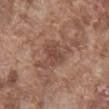Q: Was a biopsy performed?
A: no biopsy performed (imaged during a skin exam)
Q: What is the anatomic site?
A: the chest
Q: Patient demographics?
A: male, about 75 years old
Q: What is the imaging modality?
A: total-body-photography crop, ~15 mm field of view
Q: Lesion size?
A: about 5.5 mm
Q: What did automated image analysis measure?
A: a lesion area of about 12 mm², a shape eccentricity near 0.6, and a symmetry-axis asymmetry near 0.4; an average lesion color of about L≈47 a*≈20 b*≈26 (CIELAB), a lesion–skin lightness drop of about 8, and a normalized border contrast of about 6; a border-irregularity rating of about 5/10
Q: Illumination type?
A: white-light illumination